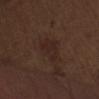Impression:
No biopsy was performed on this lesion — it was imaged during a full skin examination and was not determined to be concerning.
Acquisition and patient details:
The lesion-visualizer software estimated a lesion area of about 7.5 mm², a shape eccentricity near 0.6, and a symmetry-axis asymmetry near 0.3. The analysis additionally found a lesion color around L≈23 a*≈16 b*≈19 in CIELAB, a lesion–skin lightness drop of about 5, and a normalized border contrast of about 6. It also reported a border-irregularity rating of about 2.5/10, a color-variation rating of about 2/10, and radial color variation of about 0.5. Cropped from a whole-body photographic skin survey; the tile spans about 15 mm. About 3.5 mm across. Captured under white-light illumination. A male subject, aged 68 to 72. Located on the abdomen.